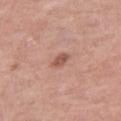Q: Was a biopsy performed?
A: no biopsy performed (imaged during a skin exam)
Q: Who is the patient?
A: female, roughly 70 years of age
Q: How was the tile lit?
A: white-light
Q: Where on the body is the lesion?
A: the right thigh
Q: How was this image acquired?
A: total-body-photography crop, ~15 mm field of view